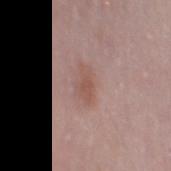Part of a total-body skin-imaging series; this lesion was reviewed on a skin check and was not flagged for biopsy. A roughly 15 mm field-of-view crop from a total-body skin photograph. Automated image analysis of the tile measured an average lesion color of about L≈53 a*≈20 b*≈24 (CIELAB), about 7 CIELAB-L* units darker than the surrounding skin, and a normalized border contrast of about 6. It also reported a border-irregularity index near 3.5/10, internal color variation of about 2 on a 0–10 scale, and radial color variation of about 0.5. A female subject aged 28 to 32. Located on the left thigh. About 3.5 mm across.